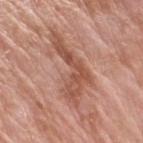biopsy_status: not biopsied; imaged during a skin examination
site: arm
patient:
  sex: male
  age_approx: 80
lesion_size:
  long_diameter_mm_approx: 9.0
image:
  source: total-body photography crop
  field_of_view_mm: 15
lighting: white-light